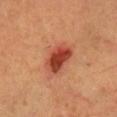Captured during whole-body skin photography for melanoma surveillance; the lesion was not biopsied. A female subject approximately 45 years of age. On the left leg. The total-body-photography lesion software estimated a footprint of about 9.5 mm², an outline eccentricity of about 0.8 (0 = round, 1 = elongated), and two-axis asymmetry of about 0.2. The analysis additionally found an average lesion color of about L≈46 a*≈33 b*≈35 (CIELAB) and roughly 14 lightness units darker than nearby skin. The software also gave a border-irregularity rating of about 2/10, a within-lesion color-variation index near 6.5/10, and a peripheral color-asymmetry measure near 2. The tile uses cross-polarized illumination. About 4.5 mm across. A lesion tile, about 15 mm wide, cut from a 3D total-body photograph.